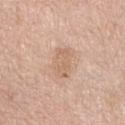Assessment: The lesion was tiled from a total-body skin photograph and was not biopsied. Context: The lesion is on the front of the torso. A female patient, aged 73 to 77. A 15 mm close-up extracted from a 3D total-body photography capture.